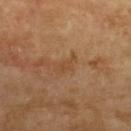location: the left forearm; acquisition: 15 mm crop, total-body photography; subject: female, aged 68 to 72.